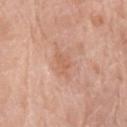Assessment:
Recorded during total-body skin imaging; not selected for excision or biopsy.
Clinical summary:
On the arm. Measured at roughly 2.5 mm in maximum diameter. A female patient, aged around 75. Cropped from a whole-body photographic skin survey; the tile spans about 15 mm.